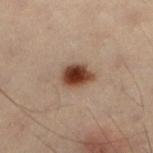Captured during whole-body skin photography for melanoma surveillance; the lesion was not biopsied. A male subject in their mid-50s. This image is a 15 mm lesion crop taken from a total-body photograph. The lesion is located on the left lower leg.